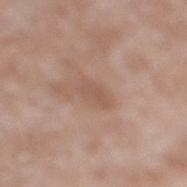follow-up: total-body-photography surveillance lesion; no biopsy
tile lighting: white-light
automated lesion analysis: an area of roughly 4.5 mm² and an outline eccentricity of about 0.8 (0 = round, 1 = elongated); a lesion color around L≈55 a*≈18 b*≈27 in CIELAB, roughly 6 lightness units darker than nearby skin, and a normalized border contrast of about 4.5; a nevus-likeness score of about 0/100 and lesion-presence confidence of about 100/100
patient: male, roughly 60 years of age
body site: the right lower leg
lesion size: about 3 mm
image source: ~15 mm tile from a whole-body skin photo